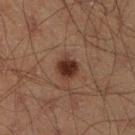This lesion was catalogued during total-body skin photography and was not selected for biopsy.